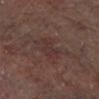subject: male, in their mid- to late 60s; anatomic site: the left lower leg; diameter: ~3 mm (longest diameter); illumination: cross-polarized; imaging modality: ~15 mm tile from a whole-body skin photo.